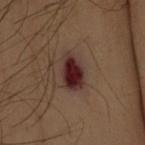Q: Illumination type?
A: cross-polarized
Q: What are the patient's age and sex?
A: female, aged 48–52
Q: Automated lesion metrics?
A: a shape eccentricity near 0.8 and a shape-asymmetry score of about 0.2 (0 = symmetric); a lesion–skin lightness drop of about 12 and a lesion-to-skin contrast of about 13.5 (normalized; higher = more distinct); border irregularity of about 2 on a 0–10 scale, a within-lesion color-variation index near 4.5/10, and a peripheral color-asymmetry measure near 1.5
Q: What is the lesion's diameter?
A: ~4 mm (longest diameter)
Q: Where on the body is the lesion?
A: the arm
Q: What is the imaging modality?
A: ~15 mm crop, total-body skin-cancer survey